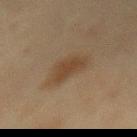Background: Cropped from a whole-body photographic skin survey; the tile spans about 15 mm. A female subject, about 55 years old. The lesion is on the mid back.